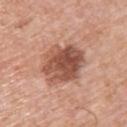Acquisition and patient details: About 6 mm across. A male subject in their 70s. A lesion tile, about 15 mm wide, cut from a 3D total-body photograph. Automated image analysis of the tile measured a footprint of about 19 mm² and an outline eccentricity of about 0.6 (0 = round, 1 = elongated). The software also gave a nevus-likeness score of about 10/100. Captured under white-light illumination. Located on the upper back.